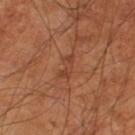Clinical impression: Captured during whole-body skin photography for melanoma surveillance; the lesion was not biopsied. Acquisition and patient details: A 15 mm close-up extracted from a 3D total-body photography capture. The recorded lesion diameter is about 3 mm. The lesion is on the leg. Automated tile analysis of the lesion measured a lesion area of about 3.5 mm², an outline eccentricity of about 0.9 (0 = round, 1 = elongated), and a shape-asymmetry score of about 0.4 (0 = symmetric). The analysis additionally found border irregularity of about 4.5 on a 0–10 scale, a color-variation rating of about 0/10, and a peripheral color-asymmetry measure near 0. It also reported a classifier nevus-likeness of about 0/100. A male subject, aged 58 to 62.